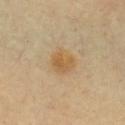Impression: Captured during whole-body skin photography for melanoma surveillance; the lesion was not biopsied. Context: A male patient in their mid- to late 50s. On the chest. This is a cross-polarized tile. The recorded lesion diameter is about 3 mm. A 15 mm close-up extracted from a 3D total-body photography capture.